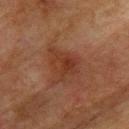The lesion was photographed on a routine skin check and not biopsied; there is no pathology result.
A 15 mm close-up extracted from a 3D total-body photography capture.
From the upper back.
A male patient aged around 75.